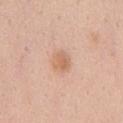Part of a total-body skin-imaging series; this lesion was reviewed on a skin check and was not flagged for biopsy. The lesion's longest dimension is about 2.5 mm. On the chest. The total-body-photography lesion software estimated a lesion color around L≈65 a*≈21 b*≈33 in CIELAB, a lesion–skin lightness drop of about 9, and a normalized lesion–skin contrast near 6.5. The analysis additionally found an automated nevus-likeness rating near 35 out of 100 and a detector confidence of about 100 out of 100 that the crop contains a lesion. This image is a 15 mm lesion crop taken from a total-body photograph. Captured under white-light illumination. The subject is a male aged 33 to 37.